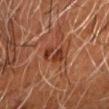Findings:
- biopsy status — total-body-photography surveillance lesion; no biopsy
- subject — male, aged 58–62
- location — the head or neck
- image — ~15 mm crop, total-body skin-cancer survey